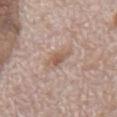Case summary:
• follow-up: imaged on a skin check; not biopsied
• automated metrics: roughly 8 lightness units darker than nearby skin; a border-irregularity rating of about 3/10, a within-lesion color-variation index near 3.5/10, and peripheral color asymmetry of about 1
• acquisition: ~15 mm crop, total-body skin-cancer survey
• lighting: white-light illumination
• anatomic site: the back
• subject: male, in their 70s
• lesion diameter: about 3 mm